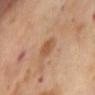Recorded during total-body skin imaging; not selected for excision or biopsy.
Imaged with cross-polarized lighting.
Located on the abdomen.
A female patient aged 53–57.
A 15 mm crop from a total-body photograph taken for skin-cancer surveillance.
About 3 mm across.